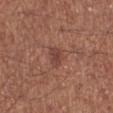Impression:
Imaged during a routine full-body skin examination; the lesion was not biopsied and no histopathology is available.
Image and clinical context:
Measured at roughly 2.5 mm in maximum diameter. Cropped from a total-body skin-imaging series; the visible field is about 15 mm. The lesion is located on the leg. Imaged with white-light lighting. A male subject approximately 70 years of age. The lesion-visualizer software estimated an outline eccentricity of about 0.55 (0 = round, 1 = elongated) and two-axis asymmetry of about 0.35. It also reported a border-irregularity rating of about 3/10, a color-variation rating of about 3/10, and peripheral color asymmetry of about 1.